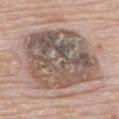No biopsy was performed on this lesion — it was imaged during a full skin examination and was not determined to be concerning.